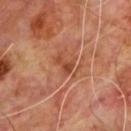{"biopsy_status": "not biopsied; imaged during a skin examination", "automated_metrics": {"area_mm2_approx": 3.0, "eccentricity": 0.8, "border_irregularity_0_10": 5.0, "peripheral_color_asymmetry": 0.0, "lesion_detection_confidence_0_100": 100}, "image": {"source": "total-body photography crop", "field_of_view_mm": 15}, "site": "back", "patient": {"sex": "male", "age_approx": 60}}